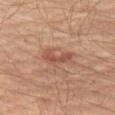No biopsy was performed on this lesion — it was imaged during a full skin examination and was not determined to be concerning. A 15 mm crop from a total-body photograph taken for skin-cancer surveillance. The lesion is on the left thigh. Measured at roughly 4 mm in maximum diameter. A male subject, roughly 65 years of age.